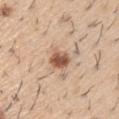The lesion was photographed on a routine skin check and not biopsied; there is no pathology result.
Cropped from a total-body skin-imaging series; the visible field is about 15 mm.
Located on the right upper arm.
The tile uses white-light illumination.
Longest diameter approximately 2.5 mm.
An algorithmic analysis of the crop reported a border-irregularity index near 2/10, internal color variation of about 3.5 on a 0–10 scale, and a peripheral color-asymmetry measure near 1. The software also gave a detector confidence of about 100 out of 100 that the crop contains a lesion.
The patient is a male aged 58–62.